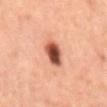<case>
  <biopsy_status>not biopsied; imaged during a skin examination</biopsy_status>
  <patient>
    <sex>male</sex>
    <age_approx>55</age_approx>
  </patient>
  <image>
    <source>total-body photography crop</source>
    <field_of_view_mm>15</field_of_view_mm>
  </image>
  <lighting>cross-polarized</lighting>
  <site>mid back</site>
  <lesion_size>
    <long_diameter_mm_approx>3.5</long_diameter_mm_approx>
  </lesion_size>
</case>